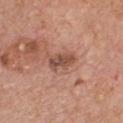{
  "biopsy_status": "not biopsied; imaged during a skin examination",
  "image": {
    "source": "total-body photography crop",
    "field_of_view_mm": 15
  },
  "lesion_size": {
    "long_diameter_mm_approx": 3.5
  },
  "patient": {
    "sex": "male",
    "age_approx": 60
  },
  "site": "chest",
  "lighting": "white-light",
  "automated_metrics": {
    "area_mm2_approx": 5.5,
    "eccentricity": 0.85
  }
}